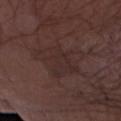A lesion tile, about 15 mm wide, cut from a 3D total-body photograph. A male subject, aged 73 to 77. On the arm.